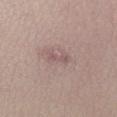Assessment: This lesion was catalogued during total-body skin photography and was not selected for biopsy. Acquisition and patient details: Longest diameter approximately 2.5 mm. An algorithmic analysis of the crop reported a footprint of about 2.5 mm², an outline eccentricity of about 0.9 (0 = round, 1 = elongated), and two-axis asymmetry of about 0.4. And it measured a mean CIELAB color near L≈54 a*≈19 b*≈18, roughly 7 lightness units darker than nearby skin, and a normalized lesion–skin contrast near 5.5. The software also gave a classifier nevus-likeness of about 0/100 and a lesion-detection confidence of about 95/100. A female patient roughly 25 years of age. The tile uses white-light illumination. Cropped from a total-body skin-imaging series; the visible field is about 15 mm. The lesion is located on the left lower leg.